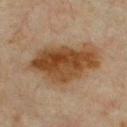Captured during whole-body skin photography for melanoma surveillance; the lesion was not biopsied.
A region of skin cropped from a whole-body photographic capture, roughly 15 mm wide.
Located on the upper back.
A male patient in their mid- to late 60s.
Imaged with cross-polarized lighting.
Longest diameter approximately 8.5 mm.